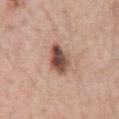Recorded during total-body skin imaging; not selected for excision or biopsy.
Approximately 4 mm at its widest.
A 15 mm close-up extracted from a 3D total-body photography capture.
The lesion is on the chest.
The total-body-photography lesion software estimated a lesion area of about 8.5 mm² and a shape eccentricity near 0.75.
A male patient approximately 60 years of age.